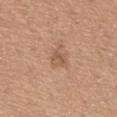Clinical impression:
Captured during whole-body skin photography for melanoma surveillance; the lesion was not biopsied.
Image and clinical context:
On the mid back. This image is a 15 mm lesion crop taken from a total-body photograph. A male patient in their mid-60s. Approximately 2.5 mm at its widest. This is a white-light tile. Automated image analysis of the tile measured an average lesion color of about L≈56 a*≈19 b*≈32 (CIELAB). It also reported a border-irregularity index near 3.5/10, a color-variation rating of about 3/10, and radial color variation of about 1.